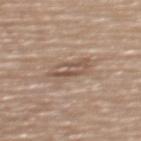Findings:
- workup · total-body-photography surveillance lesion; no biopsy
- lesion size · about 4.5 mm
- illumination · white-light illumination
- automated metrics · a footprint of about 9 mm² and a symmetry-axis asymmetry near 0.2; a border-irregularity index near 2.5/10, internal color variation of about 7 on a 0–10 scale, and radial color variation of about 2.5; a classifier nevus-likeness of about 0/100 and a lesion-detection confidence of about 75/100
- body site · the back
- acquisition · 15 mm crop, total-body photography
- subject · female, in their mid- to late 70s